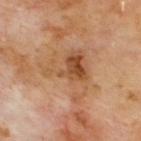No biopsy was performed on this lesion — it was imaged during a full skin examination and was not determined to be concerning.
Measured at roughly 6 mm in maximum diameter.
Imaged with cross-polarized lighting.
On the upper back.
This image is a 15 mm lesion crop taken from a total-body photograph.
A male subject aged 68–72.